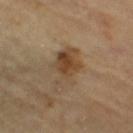Impression: Imaged during a routine full-body skin examination; the lesion was not biopsied and no histopathology is available. Image and clinical context: Imaged with cross-polarized lighting. A close-up tile cropped from a whole-body skin photograph, about 15 mm across. The patient is in their 60s. The lesion is on the right thigh.